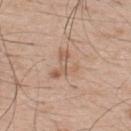follow-up: catalogued during a skin exam; not biopsied | site: the back | subject: male, aged approximately 50 | lesion diameter: about 4 mm | image: ~15 mm crop, total-body skin-cancer survey.